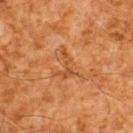Clinical impression: Recorded during total-body skin imaging; not selected for excision or biopsy. Image and clinical context: A male subject aged around 60. Approximately 3.5 mm at its widest. The lesion is on the upper back. This image is a 15 mm lesion crop taken from a total-body photograph. The lesion-visualizer software estimated a border-irregularity rating of about 8/10 and internal color variation of about 0 on a 0–10 scale. It also reported an automated nevus-likeness rating near 0 out of 100 and lesion-presence confidence of about 100/100. This is a cross-polarized tile.